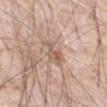size=≈4 mm | automated lesion analysis=a lesion color around L≈59 a*≈17 b*≈28 in CIELAB and roughly 10 lightness units darker than nearby skin | patient=male, roughly 80 years of age | image source=15 mm crop, total-body photography | location=the front of the torso | illumination=white-light illumination.